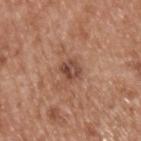Assessment:
The lesion was tiled from a total-body skin photograph and was not biopsied.
Clinical summary:
A lesion tile, about 15 mm wide, cut from a 3D total-body photograph. The lesion is located on the upper back. The subject is a male about 65 years old.